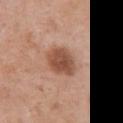  biopsy_status: not biopsied; imaged during a skin examination
  site: chest
  lighting: white-light
  automated_metrics:
    area_mm2_approx: 10.0
    eccentricity: 0.6
    border_irregularity_0_10: 1.0
    color_variation_0_10: 4.0
    peripheral_color_asymmetry: 1.5
    lesion_detection_confidence_0_100: 100
  image:
    source: total-body photography crop
    field_of_view_mm: 15
  patient:
    sex: female
    age_approx: 50